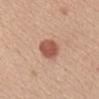follow-up: catalogued during a skin exam; not biopsied
subject: female, aged 38 to 42
acquisition: 15 mm crop, total-body photography
location: the chest
lesion diameter: ~3.5 mm (longest diameter)
lighting: white-light illumination
image-analysis metrics: a footprint of about 7 mm², an eccentricity of roughly 0.75, and a symmetry-axis asymmetry near 0.25; an average lesion color of about L≈54 a*≈26 b*≈29 (CIELAB) and about 14 CIELAB-L* units darker than the surrounding skin; a border-irregularity rating of about 2/10 and radial color variation of about 1; an automated nevus-likeness rating near 100 out of 100 and a lesion-detection confidence of about 100/100